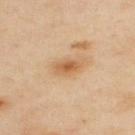Assessment: Imaged during a routine full-body skin examination; the lesion was not biopsied and no histopathology is available. Clinical summary: Measured at roughly 3 mm in maximum diameter. Imaged with cross-polarized lighting. A male subject aged around 55. A roughly 15 mm field-of-view crop from a total-body skin photograph. The lesion is located on the upper back. An algorithmic analysis of the crop reported a footprint of about 5 mm², a shape eccentricity near 0.8, and a shape-asymmetry score of about 0.2 (0 = symmetric). The software also gave a border-irregularity rating of about 1.5/10, a within-lesion color-variation index near 4.5/10, and a peripheral color-asymmetry measure near 1.5. The software also gave a nevus-likeness score of about 65/100 and a lesion-detection confidence of about 100/100.